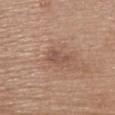Case summary:
- biopsy status — catalogued during a skin exam; not biopsied
- image — 15 mm crop, total-body photography
- site — the upper back
- subject — female, aged approximately 65
- lesion size — about 3 mm
- lighting — white-light illumination